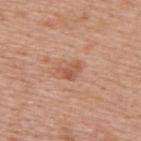{"patient": {"sex": "male", "age_approx": 75}, "site": "upper back", "automated_metrics": {"cielab_L": 56, "cielab_a": 24, "cielab_b": 33, "vs_skin_darker_L": 9.0, "vs_skin_contrast_norm": 6.5, "border_irregularity_0_10": 4.0, "color_variation_0_10": 3.0, "peripheral_color_asymmetry": 1.0, "lesion_detection_confidence_0_100": 100}, "image": {"source": "total-body photography crop", "field_of_view_mm": 15}, "lesion_size": {"long_diameter_mm_approx": 3.0}}